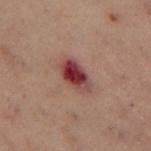Imaged during a routine full-body skin examination; the lesion was not biopsied and no histopathology is available.
About 4 mm across.
The subject is a female aged 53 to 57.
An algorithmic analysis of the crop reported about 13 CIELAB-L* units darker than the surrounding skin and a lesion-to-skin contrast of about 12 (normalized; higher = more distinct). The analysis additionally found a border-irregularity index near 2/10, a color-variation rating of about 8.5/10, and radial color variation of about 2.5. The software also gave a classifier nevus-likeness of about 0/100 and a lesion-detection confidence of about 100/100.
Cropped from a whole-body photographic skin survey; the tile spans about 15 mm.
On the left thigh.
Imaged with cross-polarized lighting.